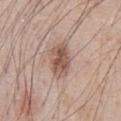No biopsy was performed on this lesion — it was imaged during a full skin examination and was not determined to be concerning.
The tile uses white-light illumination.
Measured at roughly 4.5 mm in maximum diameter.
The patient is a male aged approximately 70.
A roughly 15 mm field-of-view crop from a total-body skin photograph.
Automated image analysis of the tile measured a mean CIELAB color near L≈55 a*≈18 b*≈25 and a normalized border contrast of about 8.5.
From the chest.